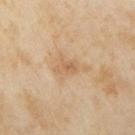Assessment:
The lesion was photographed on a routine skin check and not biopsied; there is no pathology result.
Image and clinical context:
Cropped from a whole-body photographic skin survey; the tile spans about 15 mm. This is a cross-polarized tile. Located on the right upper arm. The patient is a female approximately 35 years of age. An algorithmic analysis of the crop reported an area of roughly 3.5 mm², a shape eccentricity near 0.8, and two-axis asymmetry of about 0.3. Approximately 2.5 mm at its widest.